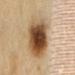<lesion>
<biopsy_status>not biopsied; imaged during a skin examination</biopsy_status>
<lighting>cross-polarized</lighting>
<site>lower back</site>
<image>
  <source>total-body photography crop</source>
  <field_of_view_mm>15</field_of_view_mm>
</image>
<patient>
  <sex>female</sex>
  <age_approx>60</age_approx>
</patient>
<lesion_size>
  <long_diameter_mm_approx>7.5</long_diameter_mm_approx>
</lesion_size>
<automated_metrics>
  <area_mm2_approx>28.0</area_mm2_approx>
  <eccentricity>0.75</eccentricity>
  <nevus_likeness_0_100>100</nevus_likeness_0_100>
  <lesion_detection_confidence_0_100>100</lesion_detection_confidence_0_100>
</automated_metrics>
</lesion>